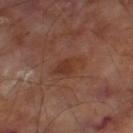Assessment:
Captured during whole-body skin photography for melanoma surveillance; the lesion was not biopsied.
Context:
Longest diameter approximately 3.5 mm. The subject is a male aged around 70. The lesion is located on the left thigh. An algorithmic analysis of the crop reported a normalized border contrast of about 6. And it measured a border-irregularity index near 3.5/10 and a within-lesion color-variation index near 2/10. And it measured a classifier nevus-likeness of about 0/100 and a detector confidence of about 100 out of 100 that the crop contains a lesion. A 15 mm crop from a total-body photograph taken for skin-cancer surveillance. Imaged with cross-polarized lighting.